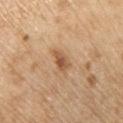The lesion was tiled from a total-body skin photograph and was not biopsied. A 15 mm close-up extracted from a 3D total-body photography capture. A male subject approximately 70 years of age. Located on the front of the torso. Measured at roughly 3 mm in maximum diameter. An algorithmic analysis of the crop reported an area of roughly 4 mm², a shape eccentricity near 0.85, and two-axis asymmetry of about 0.25. It also reported a lesion–skin lightness drop of about 11.